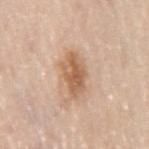The total-body-photography lesion software estimated an average lesion color of about L≈61 a*≈19 b*≈33 (CIELAB), a lesion–skin lightness drop of about 12, and a normalized lesion–skin contrast near 8. The analysis additionally found internal color variation of about 4.5 on a 0–10 scale. And it measured a classifier nevus-likeness of about 75/100 and lesion-presence confidence of about 100/100.
A female patient aged 58 to 62.
The lesion is on the right thigh.
This image is a 15 mm lesion crop taken from a total-body photograph.